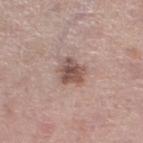Captured during whole-body skin photography for melanoma surveillance; the lesion was not biopsied. An algorithmic analysis of the crop reported an area of roughly 7.5 mm² and a symmetry-axis asymmetry near 0.25. It also reported lesion-presence confidence of about 100/100. This is a white-light tile. On the left lower leg. Cropped from a whole-body photographic skin survey; the tile spans about 15 mm. The patient is a female approximately 65 years of age.